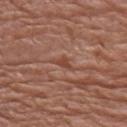Captured during whole-body skin photography for melanoma surveillance; the lesion was not biopsied.
The lesion is on the right upper arm.
Longest diameter approximately 3 mm.
This image is a 15 mm lesion crop taken from a total-body photograph.
Imaged with white-light lighting.
A female subject, roughly 80 years of age.